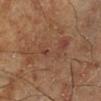<case>
<biopsy_status>not biopsied; imaged during a skin examination</biopsy_status>
<image>
  <source>total-body photography crop</source>
  <field_of_view_mm>15</field_of_view_mm>
</image>
<automated_metrics>
  <area_mm2_approx>12.0</area_mm2_approx>
  <eccentricity>0.95</eccentricity>
  <cielab_L>32</cielab_L>
  <cielab_a>16</cielab_a>
  <cielab_b>22</cielab_b>
  <vs_skin_darker_L>5.0</vs_skin_darker_L>
  <vs_skin_contrast_norm>5.0</vs_skin_contrast_norm>
  <lesion_detection_confidence_0_100>80</lesion_detection_confidence_0_100>
</automated_metrics>
<lesion_size>
  <long_diameter_mm_approx>8.0</long_diameter_mm_approx>
</lesion_size>
<site>left lower leg</site>
<patient>
  <sex>male</sex>
  <age_approx>60</age_approx>
</patient>
<lighting>cross-polarized</lighting>
</case>